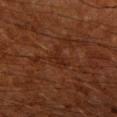Q: Was a biopsy performed?
A: total-body-photography surveillance lesion; no biopsy
Q: Lesion size?
A: about 3 mm
Q: Lesion location?
A: the arm
Q: What kind of image is this?
A: ~15 mm tile from a whole-body skin photo
Q: Automated lesion metrics?
A: a footprint of about 5 mm², a shape eccentricity near 0.6, and a shape-asymmetry score of about 0.5 (0 = symmetric); an average lesion color of about L≈21 a*≈20 b*≈25 (CIELAB), roughly 4 lightness units darker than nearby skin, and a normalized border contrast of about 5
Q: Who is the patient?
A: male, approximately 60 years of age
Q: What lighting was used for the tile?
A: cross-polarized illumination